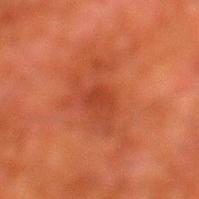workup: no biopsy performed (imaged during a skin exam) | tile lighting: cross-polarized illumination | image source: ~15 mm crop, total-body skin-cancer survey | lesion diameter: ~2.5 mm (longest diameter) | patient: male, approximately 80 years of age | body site: the left lower leg.